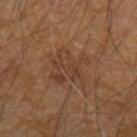<case>
  <biopsy_status>not biopsied; imaged during a skin examination</biopsy_status>
  <patient>
    <sex>male</sex>
    <age_approx>65</age_approx>
  </patient>
  <site>left upper arm</site>
  <automated_metrics>
    <eccentricity>0.55</eccentricity>
    <shape_asymmetry>0.35</shape_asymmetry>
  </automated_metrics>
  <lighting>cross-polarized</lighting>
  <lesion_size>
    <long_diameter_mm_approx>5.0</long_diameter_mm_approx>
  </lesion_size>
  <image>
    <source>total-body photography crop</source>
    <field_of_view_mm>15</field_of_view_mm>
  </image>
</case>